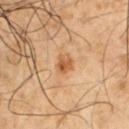Part of a total-body skin-imaging series; this lesion was reviewed on a skin check and was not flagged for biopsy. A 15 mm crop from a total-body photograph taken for skin-cancer surveillance. A male subject aged 48–52. The lesion's longest dimension is about 2.5 mm. Located on the left upper arm.